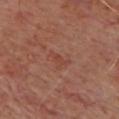Recorded during total-body skin imaging; not selected for excision or biopsy. Imaged with cross-polarized lighting. A lesion tile, about 15 mm wide, cut from a 3D total-body photograph. A male subject, aged 63–67. From the chest. The lesion-visualizer software estimated a border-irregularity index near 5.5/10, internal color variation of about 0 on a 0–10 scale, and radial color variation of about 0. The lesion's longest dimension is about 3 mm.